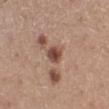Assessment:
The lesion was photographed on a routine skin check and not biopsied; there is no pathology result.
Context:
The lesion is located on the left lower leg. A roughly 15 mm field-of-view crop from a total-body skin photograph. A female subject about 45 years old. Automated image analysis of the tile measured a footprint of about 4.5 mm², an outline eccentricity of about 0.45 (0 = round, 1 = elongated), and a shape-asymmetry score of about 0.25 (0 = symmetric). It also reported border irregularity of about 2 on a 0–10 scale and peripheral color asymmetry of about 2. And it measured a nevus-likeness score of about 75/100 and a lesion-detection confidence of about 100/100.